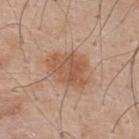biopsy status: catalogued during a skin exam; not biopsied | illumination: white-light | TBP lesion metrics: a footprint of about 13 mm², a shape eccentricity near 0.7, and a symmetry-axis asymmetry near 0.3; a nevus-likeness score of about 65/100 and a detector confidence of about 100 out of 100 that the crop contains a lesion | acquisition: 15 mm crop, total-body photography | patient: male, roughly 50 years of age | site: the upper back.